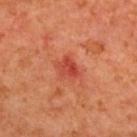biopsy status: total-body-photography surveillance lesion; no biopsy | image: total-body-photography crop, ~15 mm field of view | anatomic site: the upper back | diameter: about 2.5 mm | subject: female, roughly 40 years of age | lighting: cross-polarized illumination.